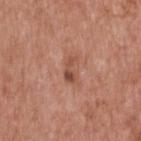Recorded during total-body skin imaging; not selected for excision or biopsy.
A 15 mm close-up tile from a total-body photography series done for melanoma screening.
Located on the upper back.
The subject is a male approximately 60 years of age.
The lesion-visualizer software estimated a footprint of about 3 mm², an outline eccentricity of about 0.9 (0 = round, 1 = elongated), and a shape-asymmetry score of about 0.5 (0 = symmetric). And it measured a mean CIELAB color near L≈49 a*≈24 b*≈30 and about 10 CIELAB-L* units darker than the surrounding skin. And it measured a within-lesion color-variation index near 2/10 and a peripheral color-asymmetry measure near 1.
The lesion's longest dimension is about 3 mm.